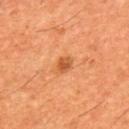Captured during whole-body skin photography for melanoma surveillance; the lesion was not biopsied.
This image is a 15 mm lesion crop taken from a total-body photograph.
The patient is a male about 60 years old.
On the mid back.
The recorded lesion diameter is about 2 mm.
The total-body-photography lesion software estimated a lesion area of about 3 mm², an eccentricity of roughly 0.6, and two-axis asymmetry of about 0.2. It also reported a nevus-likeness score of about 85/100.